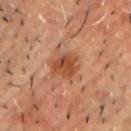| feature | finding |
|---|---|
| notes | total-body-photography surveillance lesion; no biopsy |
| diameter | about 3 mm |
| anatomic site | the chest |
| image source | ~15 mm crop, total-body skin-cancer survey |
| lighting | cross-polarized illumination |
| subject | male, aged around 50 |